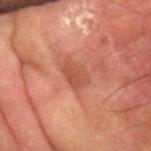No biopsy was performed on this lesion — it was imaged during a full skin examination and was not determined to be concerning.
A region of skin cropped from a whole-body photographic capture, roughly 15 mm wide.
Measured at roughly 3.5 mm in maximum diameter.
The patient is a male approximately 80 years of age.
From the left forearm.
The lesion-visualizer software estimated an area of roughly 6 mm² and two-axis asymmetry of about 0.25.